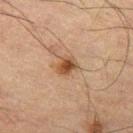Assessment:
Captured during whole-body skin photography for melanoma surveillance; the lesion was not biopsied.
Background:
Measured at roughly 2.5 mm in maximum diameter. The tile uses cross-polarized illumination. A 15 mm crop from a total-body photograph taken for skin-cancer surveillance. A male subject, about 65 years old. On the left thigh.